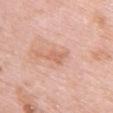Clinical impression: Imaged during a routine full-body skin examination; the lesion was not biopsied and no histopathology is available. Context: The lesion is on the upper back. A female subject in their mid- to late 60s. A close-up tile cropped from a whole-body skin photograph, about 15 mm across.